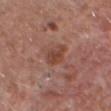| key | value |
|---|---|
| notes | total-body-photography surveillance lesion; no biopsy |
| body site | the head or neck |
| automated lesion analysis | a border-irregularity index near 2.5/10, internal color variation of about 3.5 on a 0–10 scale, and a peripheral color-asymmetry measure near 1.5; an automated nevus-likeness rating near 15 out of 100 and a detector confidence of about 100 out of 100 that the crop contains a lesion |
| illumination | white-light |
| patient | male, roughly 65 years of age |
| acquisition | ~15 mm tile from a whole-body skin photo |